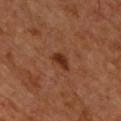– notes: no biopsy performed (imaged during a skin exam)
– acquisition: ~15 mm crop, total-body skin-cancer survey
– subject: male, aged 48 to 52
– site: the chest
– automated metrics: border irregularity of about 3 on a 0–10 scale, internal color variation of about 1.5 on a 0–10 scale, and peripheral color asymmetry of about 0.5; lesion-presence confidence of about 100/100